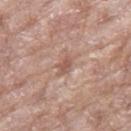The lesion was tiled from a total-body skin photograph and was not biopsied. On the right thigh. The subject is a female roughly 75 years of age. This is a white-light tile. The recorded lesion diameter is about 2.5 mm. A 15 mm close-up tile from a total-body photography series done for melanoma screening.